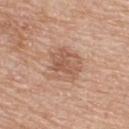Imaged during a routine full-body skin examination; the lesion was not biopsied and no histopathology is available. The tile uses white-light illumination. The lesion is located on the upper back. An algorithmic analysis of the crop reported border irregularity of about 3.5 on a 0–10 scale and a color-variation rating of about 4/10. The patient is a male aged 58–62. Cropped from a whole-body photographic skin survey; the tile spans about 15 mm.